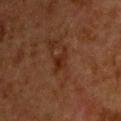<lesion>
  <biopsy_status>not biopsied; imaged during a skin examination</biopsy_status>
  <site>back</site>
  <image>
    <source>total-body photography crop</source>
    <field_of_view_mm>15</field_of_view_mm>
  </image>
  <patient>
    <sex>male</sex>
    <age_approx>65</age_approx>
  </patient>
</lesion>